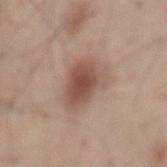| key | value |
|---|---|
| notes | no biopsy performed (imaged during a skin exam) |
| illumination | white-light |
| anatomic site | the back |
| subject | male, approximately 55 years of age |
| acquisition | ~15 mm crop, total-body skin-cancer survey |